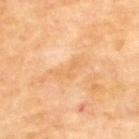The lesion was tiled from a total-body skin photograph and was not biopsied. Automated tile analysis of the lesion measured about 5 CIELAB-L* units darker than the surrounding skin. The analysis additionally found a nevus-likeness score of about 0/100 and lesion-presence confidence of about 100/100. A lesion tile, about 15 mm wide, cut from a 3D total-body photograph. Located on the upper back. Imaged with cross-polarized lighting. The subject is a male aged around 70.